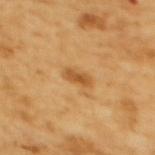* notes: no biopsy performed (imaged during a skin exam)